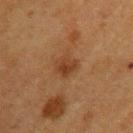Clinical impression: The lesion was tiled from a total-body skin photograph and was not biopsied. Context: This image is a 15 mm lesion crop taken from a total-body photograph. The total-body-photography lesion software estimated a lesion area of about 5.5 mm² and two-axis asymmetry of about 0.15. The software also gave lesion-presence confidence of about 100/100. From the left upper arm. This is a cross-polarized tile. The recorded lesion diameter is about 3 mm. The subject is a female aged 53 to 57.